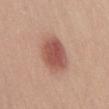Findings:
- notes · no biopsy performed (imaged during a skin exam)
- automated metrics · a lesion–skin lightness drop of about 13 and a lesion-to-skin contrast of about 8.5 (normalized; higher = more distinct)
- lesion diameter · ~4.5 mm (longest diameter)
- tile lighting · white-light
- location · the front of the torso
- acquisition · ~15 mm tile from a whole-body skin photo
- subject · male, approximately 30 years of age